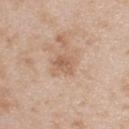Q: Was a biopsy performed?
A: no biopsy performed (imaged during a skin exam)